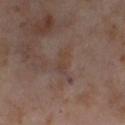diameter: ≈3 mm | lighting: cross-polarized | subject: female, in their mid- to late 50s | location: the leg | image source: ~15 mm crop, total-body skin-cancer survey.